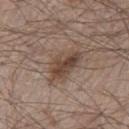Clinical impression:
The lesion was tiled from a total-body skin photograph and was not biopsied.
Acquisition and patient details:
Measured at roughly 4.5 mm in maximum diameter. On the chest. The patient is a male in their 80s. Captured under white-light illumination. Cropped from a whole-body photographic skin survey; the tile spans about 15 mm.